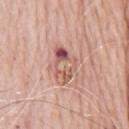Part of a total-body skin-imaging series; this lesion was reviewed on a skin check and was not flagged for biopsy.
The recorded lesion diameter is about 4.5 mm.
A male subject approximately 80 years of age.
The lesion is on the chest.
A lesion tile, about 15 mm wide, cut from a 3D total-body photograph.
This is a white-light tile.